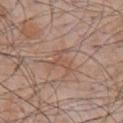biopsy_status: not biopsied; imaged during a skin examination
site: chest
patient:
  sex: male
  age_approx: 55
lesion_size:
  long_diameter_mm_approx: 3.5
image:
  source: total-body photography crop
  field_of_view_mm: 15
lighting: white-light